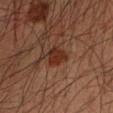follow-up: imaged on a skin check; not biopsied
size: about 3 mm
TBP lesion metrics: a lesion color around L≈28 a*≈21 b*≈26 in CIELAB, about 8 CIELAB-L* units darker than the surrounding skin, and a lesion-to-skin contrast of about 9 (normalized; higher = more distinct)
patient: male, aged 33–37
imaging modality: total-body-photography crop, ~15 mm field of view
location: the arm
lighting: cross-polarized illumination